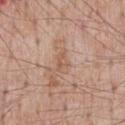Impression: Recorded during total-body skin imaging; not selected for excision or biopsy. Background: A male subject, about 55 years old. A 15 mm crop from a total-body photograph taken for skin-cancer surveillance. On the chest.